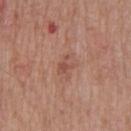follow-up: total-body-photography surveillance lesion; no biopsy
image: total-body-photography crop, ~15 mm field of view
body site: the chest
lesion diameter: ~2.5 mm (longest diameter)
subject: male, roughly 65 years of age
image-analysis metrics: a lesion area of about 3 mm², an eccentricity of roughly 0.8, and a symmetry-axis asymmetry near 0.55; a lesion color around L≈50 a*≈23 b*≈27 in CIELAB, roughly 8 lightness units darker than nearby skin, and a normalized lesion–skin contrast near 5.5; border irregularity of about 6.5 on a 0–10 scale, internal color variation of about 0 on a 0–10 scale, and radial color variation of about 0
lighting: white-light